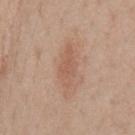notes: no biopsy performed (imaged during a skin exam)
imaging modality: total-body-photography crop, ~15 mm field of view
automated metrics: an area of roughly 7.5 mm² and a shape-asymmetry score of about 0.25 (0 = symmetric); an automated nevus-likeness rating near 0 out of 100
patient: male, roughly 55 years of age
lesion size: ≈4.5 mm
location: the chest
tile lighting: white-light illumination